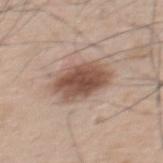• notes — imaged on a skin check; not biopsied
• imaging modality — total-body-photography crop, ~15 mm field of view
• site — the upper back
• lighting — white-light
• patient — male, approximately 65 years of age